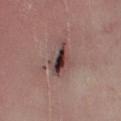Q: Is there a histopathology result?
A: total-body-photography surveillance lesion; no biopsy
Q: What is the imaging modality?
A: ~15 mm tile from a whole-body skin photo
Q: Lesion location?
A: the left lower leg
Q: Patient demographics?
A: male, aged 68 to 72